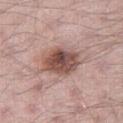Recorded during total-body skin imaging; not selected for excision or biopsy.
A 15 mm crop from a total-body photograph taken for skin-cancer surveillance.
The tile uses white-light illumination.
Longest diameter approximately 4.5 mm.
From the leg.
The subject is a male aged 38 to 42.